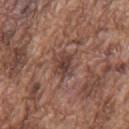Recorded during total-body skin imaging; not selected for excision or biopsy.
A male subject roughly 75 years of age.
A close-up tile cropped from a whole-body skin photograph, about 15 mm across.
On the mid back.
The recorded lesion diameter is about 3.5 mm.